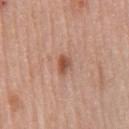Q: Automated lesion metrics?
A: a shape eccentricity near 0.75; border irregularity of about 3 on a 0–10 scale and a peripheral color-asymmetry measure near 1.5
Q: What kind of image is this?
A: ~15 mm crop, total-body skin-cancer survey
Q: Where on the body is the lesion?
A: the chest
Q: What is the lesion's diameter?
A: ~2.5 mm (longest diameter)
Q: Patient demographics?
A: male, aged 78–82